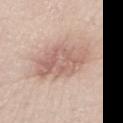Recorded during total-body skin imaging; not selected for excision or biopsy.
This image is a 15 mm lesion crop taken from a total-body photograph.
Measured at roughly 7.5 mm in maximum diameter.
From the leg.
The patient is a male roughly 80 years of age.